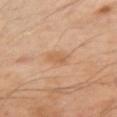Clinical impression:
Imaged during a routine full-body skin examination; the lesion was not biopsied and no histopathology is available.
Image and clinical context:
A close-up tile cropped from a whole-body skin photograph, about 15 mm across. Imaged with cross-polarized lighting. On the left arm. Measured at roughly 3 mm in maximum diameter. A male patient aged 48–52.